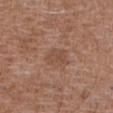Assessment: This lesion was catalogued during total-body skin photography and was not selected for biopsy. Clinical summary: From the abdomen. The subject is a male roughly 75 years of age. Cropped from a whole-body photographic skin survey; the tile spans about 15 mm. Longest diameter approximately 2.5 mm.